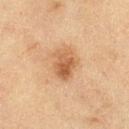Captured during whole-body skin photography for melanoma surveillance; the lesion was not biopsied. The subject is a female aged 53–57. From the left thigh. A region of skin cropped from a whole-body photographic capture, roughly 15 mm wide. The tile uses cross-polarized illumination. Automated tile analysis of the lesion measured a mean CIELAB color near L≈52 a*≈20 b*≈34, about 10 CIELAB-L* units darker than the surrounding skin, and a lesion-to-skin contrast of about 7.5 (normalized; higher = more distinct). And it measured a nevus-likeness score of about 85/100 and lesion-presence confidence of about 100/100.